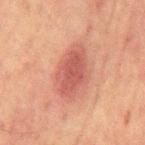No biopsy was performed on this lesion — it was imaged during a full skin examination and was not determined to be concerning. An algorithmic analysis of the crop reported an area of roughly 15 mm². The lesion is located on the front of the torso. This image is a 15 mm lesion crop taken from a total-body photograph. The lesion's longest dimension is about 6 mm. A male subject aged 63 to 67. This is a cross-polarized tile.